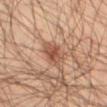Assessment: The lesion was tiled from a total-body skin photograph and was not biopsied. Background: Longest diameter approximately 4 mm. A male subject, in their mid-50s. A region of skin cropped from a whole-body photographic capture, roughly 15 mm wide. On the left thigh. Automated image analysis of the tile measured an area of roughly 7.5 mm² and a symmetry-axis asymmetry near 0.2. It also reported border irregularity of about 2.5 on a 0–10 scale, a color-variation rating of about 3.5/10, and a peripheral color-asymmetry measure near 1.